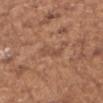biopsy status: total-body-photography surveillance lesion; no biopsy
body site: the left upper arm
image-analysis metrics: an area of roughly 4.5 mm², an outline eccentricity of about 0.9 (0 = round, 1 = elongated), and two-axis asymmetry of about 0.25; border irregularity of about 3 on a 0–10 scale, a within-lesion color-variation index near 3/10, and radial color variation of about 1; a classifier nevus-likeness of about 0/100
acquisition: ~15 mm crop, total-body skin-cancer survey
patient: female, roughly 75 years of age